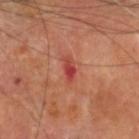follow-up: total-body-photography surveillance lesion; no biopsy | acquisition: ~15 mm crop, total-body skin-cancer survey | anatomic site: the head or neck | patient: male, aged around 70.